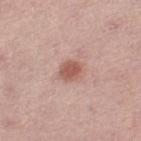<record>
<biopsy_status>not biopsied; imaged during a skin examination</biopsy_status>
<patient>
  <sex>female</sex>
  <age_approx>50</age_approx>
</patient>
<image>
  <source>total-body photography crop</source>
  <field_of_view_mm>15</field_of_view_mm>
</image>
<lesion_size>
  <long_diameter_mm_approx>3.0</long_diameter_mm_approx>
</lesion_size>
<site>left thigh</site>
</record>